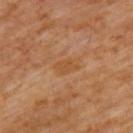Findings:
– follow-up · total-body-photography surveillance lesion; no biopsy
– site · the upper back
– imaging modality · ~15 mm crop, total-body skin-cancer survey
– patient · male, aged approximately 60